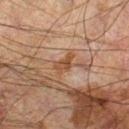Case summary:
• tile lighting · cross-polarized illumination
• patient · male, aged approximately 60
• diameter · ≈2.5 mm
• TBP lesion metrics · an area of roughly 4 mm², an outline eccentricity of about 0.75 (0 = round, 1 = elongated), and a symmetry-axis asymmetry near 0.45; an average lesion color of about L≈36 a*≈17 b*≈26 (CIELAB), a lesion–skin lightness drop of about 7, and a lesion-to-skin contrast of about 7.5 (normalized; higher = more distinct)
• acquisition · total-body-photography crop, ~15 mm field of view
• location · the left leg